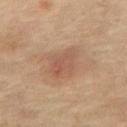Clinical impression:
Captured during whole-body skin photography for melanoma surveillance; the lesion was not biopsied.